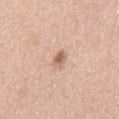This lesion was catalogued during total-body skin photography and was not selected for biopsy. On the chest. A region of skin cropped from a whole-body photographic capture, roughly 15 mm wide. A female patient roughly 40 years of age. This is a white-light tile. Approximately 2.5 mm at its widest.